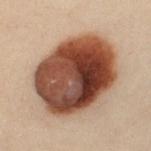• follow-up: catalogued during a skin exam; not biopsied
• automated lesion analysis: internal color variation of about 10 on a 0–10 scale and peripheral color asymmetry of about 5; a classifier nevus-likeness of about 100/100 and a lesion-detection confidence of about 100/100
• lighting: cross-polarized
• diameter: about 9.5 mm
• subject: female, about 40 years old
• image source: ~15 mm tile from a whole-body skin photo
• anatomic site: the left upper arm